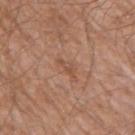biopsy status: no biopsy performed (imaged during a skin exam) | lighting: white-light | patient: male, aged 58–62 | location: the left upper arm | acquisition: 15 mm crop, total-body photography.